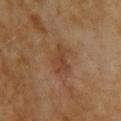Clinical impression:
Recorded during total-body skin imaging; not selected for excision or biopsy.
Background:
A female patient in their 60s. A 15 mm crop from a total-body photograph taken for skin-cancer surveillance. An algorithmic analysis of the crop reported a footprint of about 7.5 mm² and a shape-asymmetry score of about 0.25 (0 = symmetric). The software also gave a lesion color around L≈38 a*≈19 b*≈29 in CIELAB, about 6 CIELAB-L* units darker than the surrounding skin, and a normalized border contrast of about 6. And it measured a color-variation rating of about 3/10 and radial color variation of about 1. The software also gave a detector confidence of about 100 out of 100 that the crop contains a lesion. The lesion is on the upper back.